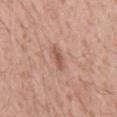Imaged during a routine full-body skin examination; the lesion was not biopsied and no histopathology is available.